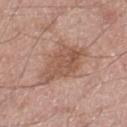The lesion was tiled from a total-body skin photograph and was not biopsied.
Cropped from a whole-body photographic skin survey; the tile spans about 15 mm.
The lesion's longest dimension is about 6 mm.
The lesion-visualizer software estimated an automated nevus-likeness rating near 15 out of 100 and a detector confidence of about 100 out of 100 that the crop contains a lesion.
Captured under white-light illumination.
The lesion is on the left thigh.
A male subject about 55 years old.